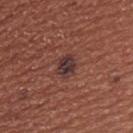workup: imaged on a skin check; not biopsied
imaging modality: 15 mm crop, total-body photography
subject: male, in their mid-40s
tile lighting: white-light
size: ~3 mm (longest diameter)
body site: the upper back
image-analysis metrics: a lesion color around L≈33 a*≈19 b*≈18 in CIELAB, about 10 CIELAB-L* units darker than the surrounding skin, and a normalized lesion–skin contrast near 11.5; a peripheral color-asymmetry measure near 1; lesion-presence confidence of about 100/100